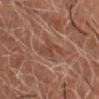Assessment:
This lesion was catalogued during total-body skin photography and was not selected for biopsy.
Context:
About 3 mm across. The lesion is on the head or neck. The subject is a male aged around 70. Imaged with cross-polarized lighting. Cropped from a total-body skin-imaging series; the visible field is about 15 mm.